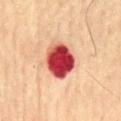The lesion was tiled from a total-body skin photograph and was not biopsied.
The lesion's longest dimension is about 4.5 mm.
The total-body-photography lesion software estimated an eccentricity of roughly 0.55 and two-axis asymmetry of about 0.15.
On the mid back.
A male patient approximately 75 years of age.
A region of skin cropped from a whole-body photographic capture, roughly 15 mm wide.